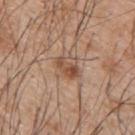Q: Was this lesion biopsied?
A: catalogued during a skin exam; not biopsied
Q: What lighting was used for the tile?
A: white-light
Q: Lesion location?
A: the upper back
Q: What did automated image analysis measure?
A: a footprint of about 7 mm² and two-axis asymmetry of about 0.2; a mean CIELAB color near L≈52 a*≈19 b*≈30 and about 9 CIELAB-L* units darker than the surrounding skin; a border-irregularity rating of about 2.5/10 and radial color variation of about 2.5
Q: Who is the patient?
A: male, aged around 50
Q: What kind of image is this?
A: 15 mm crop, total-body photography